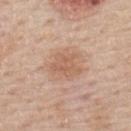follow-up: catalogued during a skin exam; not biopsied
body site: the upper back
lesion diameter: about 4 mm
imaging modality: 15 mm crop, total-body photography
tile lighting: white-light illumination
subject: male, aged approximately 60